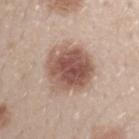Imaged during a routine full-body skin examination; the lesion was not biopsied and no histopathology is available. A male patient aged approximately 30. Cropped from a whole-body photographic skin survey; the tile spans about 15 mm. The lesion is on the left forearm. This is a white-light tile.